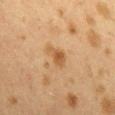follow-up=total-body-photography surveillance lesion; no biopsy | patient=female, aged 38 to 42 | image=~15 mm crop, total-body skin-cancer survey | anatomic site=the mid back | tile lighting=cross-polarized.